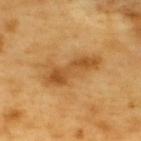Q: Is there a histopathology result?
A: total-body-photography surveillance lesion; no biopsy
Q: What is the anatomic site?
A: the back
Q: How was this image acquired?
A: ~15 mm tile from a whole-body skin photo
Q: Lesion size?
A: about 6.5 mm
Q: Patient demographics?
A: male, aged 58 to 62